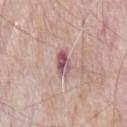<case>
  <biopsy_status>not biopsied; imaged during a skin examination</biopsy_status>
  <patient>
    <sex>male</sex>
    <age_approx>65</age_approx>
  </patient>
  <site>front of the torso</site>
  <image>
    <source>total-body photography crop</source>
    <field_of_view_mm>15</field_of_view_mm>
  </image>
</case>